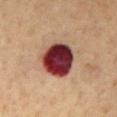{"biopsy_status": "not biopsied; imaged during a skin examination", "patient": {"sex": "male", "age_approx": 60}, "image": {"source": "total-body photography crop", "field_of_view_mm": 15}, "automated_metrics": {"area_mm2_approx": 18.0, "eccentricity": 0.55, "shape_asymmetry": 0.1, "cielab_L": 33, "cielab_a": 27, "cielab_b": 21, "vs_skin_darker_L": 24.0}, "lighting": "cross-polarized", "site": "chest", "lesion_size": {"long_diameter_mm_approx": 5.0}}